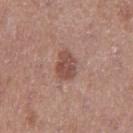Notes:
• follow-up · total-body-photography surveillance lesion; no biopsy
• acquisition · total-body-photography crop, ~15 mm field of view
• location · the leg
• size · about 3.5 mm
• patient · female, in their 40s
• lighting · white-light